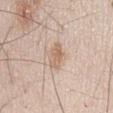biopsy_status: not biopsied; imaged during a skin examination
image:
  source: total-body photography crop
  field_of_view_mm: 15
patient:
  sex: male
  age_approx: 45
site: front of the torso